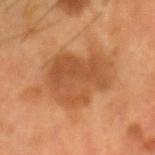Clinical impression: Captured during whole-body skin photography for melanoma surveillance; the lesion was not biopsied. Acquisition and patient details: A male subject aged 53 to 57. Cropped from a total-body skin-imaging series; the visible field is about 15 mm. The lesion is on the head or neck. Measured at roughly 7 mm in maximum diameter.